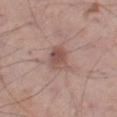  biopsy_status: not biopsied; imaged during a skin examination
  lesion_size:
    long_diameter_mm_approx: 3.0
  site: right thigh
  patient:
    sex: male
    age_approx: 70
  automated_metrics:
    area_mm2_approx: 6.5
    eccentricity: 0.6
    shape_asymmetry: 0.25
    lesion_detection_confidence_0_100: 100
  lighting: white-light
  image:
    source: total-body photography crop
    field_of_view_mm: 15